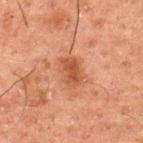follow-up: no biopsy performed (imaged during a skin exam)
patient: male, aged around 50
lighting: cross-polarized illumination
imaging modality: 15 mm crop, total-body photography
location: the upper back
lesion diameter: ≈3.5 mm